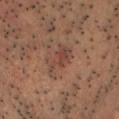Impression: Captured during whole-body skin photography for melanoma surveillance; the lesion was not biopsied. Acquisition and patient details: Located on the head or neck. Approximately 4 mm at its widest. Automated image analysis of the tile measured a mean CIELAB color near L≈43 a*≈20 b*≈25, a lesion–skin lightness drop of about 7, and a lesion-to-skin contrast of about 6 (normalized; higher = more distinct). And it measured internal color variation of about 4 on a 0–10 scale and radial color variation of about 1.5. And it measured a classifier nevus-likeness of about 5/100 and a lesion-detection confidence of about 100/100. A 15 mm crop from a total-body photograph taken for skin-cancer surveillance. A male patient, aged 63 to 67. The tile uses cross-polarized illumination.